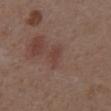No biopsy was performed on this lesion — it was imaged during a full skin examination and was not determined to be concerning.
The total-body-photography lesion software estimated a lesion color around L≈40 a*≈20 b*≈23 in CIELAB and a normalized border contrast of about 5.5. The analysis additionally found a classifier nevus-likeness of about 0/100.
Cropped from a whole-body photographic skin survey; the tile spans about 15 mm.
A male subject, in their mid-50s.
This is a white-light tile.
The recorded lesion diameter is about 2.5 mm.
Located on the abdomen.